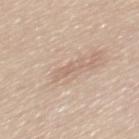<record>
<biopsy_status>not biopsied; imaged during a skin examination</biopsy_status>
<image>
  <source>total-body photography crop</source>
  <field_of_view_mm>15</field_of_view_mm>
</image>
<lesion_size>
  <long_diameter_mm_approx>3.0</long_diameter_mm_approx>
</lesion_size>
<patient>
  <sex>female</sex>
  <age_approx>55</age_approx>
</patient>
<site>mid back</site>
<lighting>white-light</lighting>
</record>